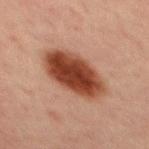Captured during whole-body skin photography for melanoma surveillance; the lesion was not biopsied.
A 15 mm close-up tile from a total-body photography series done for melanoma screening.
On the mid back.
A male subject, approximately 30 years of age.
An algorithmic analysis of the crop reported an average lesion color of about L≈36 a*≈22 b*≈27 (CIELAB), a lesion–skin lightness drop of about 15, and a normalized border contrast of about 13. And it measured a nevus-likeness score of about 100/100 and a lesion-detection confidence of about 100/100.
The tile uses cross-polarized illumination.